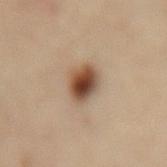No biopsy was performed on this lesion — it was imaged during a full skin examination and was not determined to be concerning. The subject is a female roughly 60 years of age. The lesion's longest dimension is about 3 mm. The lesion is on the mid back. An algorithmic analysis of the crop reported a border-irregularity index near 1.5/10, a color-variation rating of about 8/10, and peripheral color asymmetry of about 2. The software also gave a nevus-likeness score of about 100/100 and a detector confidence of about 100 out of 100 that the crop contains a lesion. Cropped from a whole-body photographic skin survey; the tile spans about 15 mm.